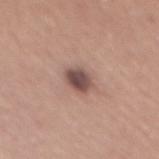Clinical impression:
Part of a total-body skin-imaging series; this lesion was reviewed on a skin check and was not flagged for biopsy.
Image and clinical context:
A roughly 15 mm field-of-view crop from a total-body skin photograph. On the mid back. The lesion's longest dimension is about 3 mm. A female subject, aged around 50.